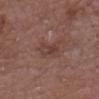No biopsy was performed on this lesion — it was imaged during a full skin examination and was not determined to be concerning.
The subject is a male aged 78–82.
From the head or neck.
A 15 mm crop from a total-body photograph taken for skin-cancer surveillance.